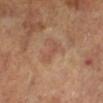• workup — total-body-photography surveillance lesion; no biopsy
• subject — female, roughly 65 years of age
• image-analysis metrics — a border-irregularity rating of about 6/10, a color-variation rating of about 0/10, and radial color variation of about 0; a classifier nevus-likeness of about 10/100 and a lesion-detection confidence of about 100/100
• acquisition — total-body-photography crop, ~15 mm field of view
• lighting — cross-polarized illumination
• anatomic site — the leg